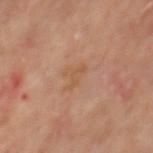A region of skin cropped from a whole-body photographic capture, roughly 15 mm wide.
The lesion is located on the mid back.
The tile uses cross-polarized illumination.
The subject is a male roughly 65 years of age.